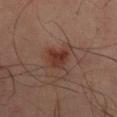The lesion was tiled from a total-body skin photograph and was not biopsied. The subject is a male aged 48–52. This image is a 15 mm lesion crop taken from a total-body photograph. The total-body-photography lesion software estimated an outline eccentricity of about 0.5 (0 = round, 1 = elongated) and two-axis asymmetry of about 0.3. It also reported a mean CIELAB color near L≈34 a*≈23 b*≈26, about 8 CIELAB-L* units darker than the surrounding skin, and a normalized lesion–skin contrast near 8.5. And it measured an automated nevus-likeness rating near 95 out of 100 and a lesion-detection confidence of about 100/100. Measured at roughly 3 mm in maximum diameter. The tile uses cross-polarized illumination. On the back.